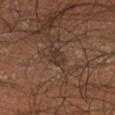Notes:
– notes: total-body-photography surveillance lesion; no biopsy
– TBP lesion metrics: a lesion color around L≈31 a*≈15 b*≈23 in CIELAB, about 7 CIELAB-L* units darker than the surrounding skin, and a normalized border contrast of about 7; internal color variation of about 3.5 on a 0–10 scale and a peripheral color-asymmetry measure near 1.5
– image: ~15 mm tile from a whole-body skin photo
– subject: male, in their mid-30s
– lesion diameter: ≈2.5 mm
– anatomic site: the right lower leg
– illumination: cross-polarized illumination